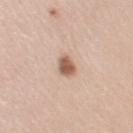Clinical impression: Recorded during total-body skin imaging; not selected for excision or biopsy. Background: A region of skin cropped from a whole-body photographic capture, roughly 15 mm wide. On the left arm. A female subject about 40 years old.